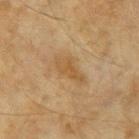biopsy_status: not biopsied; imaged during a skin examination
image:
  source: total-body photography crop
  field_of_view_mm: 15
site: right forearm
patient:
  sex: female
  age_approx: 60
lighting: cross-polarized
lesion_size:
  long_diameter_mm_approx: 4.5
automated_metrics:
  cielab_L: 49
  cielab_a: 16
  cielab_b: 36
  vs_skin_contrast_norm: 6.0
  nevus_likeness_0_100: 0